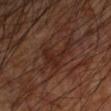This lesion was catalogued during total-body skin photography and was not selected for biopsy. Longest diameter approximately 4 mm. A roughly 15 mm field-of-view crop from a total-body skin photograph. The lesion is located on the left upper arm. This is a cross-polarized tile. A male subject in their 60s. The total-body-photography lesion software estimated an area of roughly 7 mm², a shape eccentricity near 0.7, and a shape-asymmetry score of about 0.75 (0 = symmetric). The software also gave a border-irregularity index near 8.5/10, a within-lesion color-variation index near 2/10, and a peripheral color-asymmetry measure near 0.5.